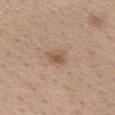Recorded during total-body skin imaging; not selected for excision or biopsy.
The tile uses white-light illumination.
The lesion is located on the head or neck.
The total-body-photography lesion software estimated a lesion area of about 3.5 mm², an eccentricity of roughly 0.75, and a symmetry-axis asymmetry near 0.3. It also reported a mean CIELAB color near L≈54 a*≈18 b*≈30 and roughly 9 lightness units darker than nearby skin. The software also gave a border-irregularity index near 2.5/10 and a within-lesion color-variation index near 2.5/10. It also reported an automated nevus-likeness rating near 25 out of 100 and a lesion-detection confidence of about 100/100.
A 15 mm close-up extracted from a 3D total-body photography capture.
The patient is a female aged around 40.